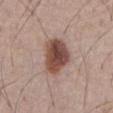<tbp_lesion>
<biopsy_status>not biopsied; imaged during a skin examination</biopsy_status>
<image>
  <source>total-body photography crop</source>
  <field_of_view_mm>15</field_of_view_mm>
</image>
<automated_metrics>
  <nevus_likeness_0_100>100</nevus_likeness_0_100>
  <lesion_detection_confidence_0_100>100</lesion_detection_confidence_0_100>
</automated_metrics>
<lesion_size>
  <long_diameter_mm_approx>5.0</long_diameter_mm_approx>
</lesion_size>
<lighting>white-light</lighting>
<patient>
  <sex>male</sex>
  <age_approx>65</age_approx>
</patient>
<site>chest</site>
</tbp_lesion>